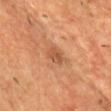Imaged during a routine full-body skin examination; the lesion was not biopsied and no histopathology is available.
The total-body-photography lesion software estimated an area of roughly 3.5 mm² and a symmetry-axis asymmetry near 0.3. And it measured a border-irregularity index near 2.5/10, a within-lesion color-variation index near 2/10, and a peripheral color-asymmetry measure near 0.5.
Located on the chest.
The patient is a male approximately 60 years of age.
The recorded lesion diameter is about 2.5 mm.
This image is a 15 mm lesion crop taken from a total-body photograph.